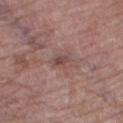Case summary:
– follow-up — total-body-photography surveillance lesion; no biopsy